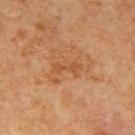notes: total-body-photography surveillance lesion; no biopsy
diameter: about 3 mm
image source: 15 mm crop, total-body photography
site: the back
subject: male, aged approximately 65
automated lesion analysis: a lesion-to-skin contrast of about 5 (normalized; higher = more distinct); a within-lesion color-variation index near 0/10 and a peripheral color-asymmetry measure near 0
illumination: cross-polarized